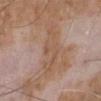{"biopsy_status": "not biopsied; imaged during a skin examination", "site": "leg", "image": {"source": "total-body photography crop", "field_of_view_mm": 15}, "lesion_size": {"long_diameter_mm_approx": 5.5}, "lighting": "white-light", "automated_metrics": {"area_mm2_approx": 10.0, "eccentricity": 0.9, "shape_asymmetry": 0.35, "vs_skin_darker_L": 6.0, "color_variation_0_10": 2.5, "peripheral_color_asymmetry": 0.5}, "patient": {"sex": "male", "age_approx": 65}}